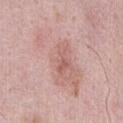Captured during whole-body skin photography for melanoma surveillance; the lesion was not biopsied.
The lesion is located on the abdomen.
A 15 mm crop from a total-body photograph taken for skin-cancer surveillance.
A male subject, aged around 60.